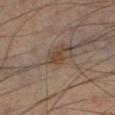Part of a total-body skin-imaging series; this lesion was reviewed on a skin check and was not flagged for biopsy. The total-body-photography lesion software estimated a footprint of about 2 mm² and a shape-asymmetry score of about 0.3 (0 = symmetric). The analysis additionally found a mean CIELAB color near L≈40 a*≈16 b*≈26, about 8 CIELAB-L* units darker than the surrounding skin, and a normalized lesion–skin contrast near 7.5. Cropped from a total-body skin-imaging series; the visible field is about 15 mm. Captured under cross-polarized illumination. A male subject, in their mid-40s. Located on the left lower leg. Longest diameter approximately 1.5 mm.